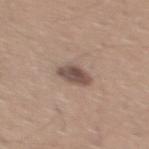workup = catalogued during a skin exam; not biopsied
subject = male, about 30 years old
image source = total-body-photography crop, ~15 mm field of view
anatomic site = the back
lighting = white-light
image-analysis metrics = an area of roughly 6.5 mm², an eccentricity of roughly 0.7, and a symmetry-axis asymmetry near 0.2; border irregularity of about 2 on a 0–10 scale, internal color variation of about 3.5 on a 0–10 scale, and peripheral color asymmetry of about 1